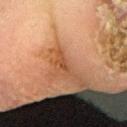Clinical summary:
A male patient, in their mid- to late 60s. Approximately 3 mm at its widest. An algorithmic analysis of the crop reported a mean CIELAB color near L≈39 a*≈21 b*≈31 and a lesion–skin lightness drop of about 8. It also reported border irregularity of about 6.5 on a 0–10 scale, a within-lesion color-variation index near 0/10, and peripheral color asymmetry of about 0. This is a cross-polarized tile. A 15 mm close-up extracted from a 3D total-body photography capture. From the right forearm.